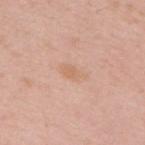The lesion was photographed on a routine skin check and not biopsied; there is no pathology result.
A region of skin cropped from a whole-body photographic capture, roughly 15 mm wide.
The tile uses white-light illumination.
From the upper back.
A female subject, aged around 40.